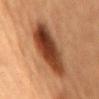follow-up: catalogued during a skin exam; not biopsied | location: the mid back | subject: female, roughly 60 years of age | imaging modality: 15 mm crop, total-body photography.